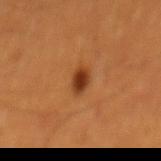Imaged during a routine full-body skin examination; the lesion was not biopsied and no histopathology is available. Cropped from a whole-body photographic skin survey; the tile spans about 15 mm. The lesion is located on the mid back. A male subject approximately 60 years of age.